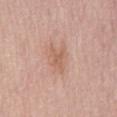Q: Was a biopsy performed?
A: no biopsy performed (imaged during a skin exam)
Q: What are the patient's age and sex?
A: male, aged 53 to 57
Q: How was this image acquired?
A: total-body-photography crop, ~15 mm field of view
Q: What is the lesion's diameter?
A: ~3.5 mm (longest diameter)
Q: How was the tile lit?
A: white-light illumination